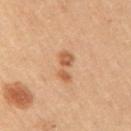Impression: The lesion was tiled from a total-body skin photograph and was not biopsied. Clinical summary: This is a cross-polarized tile. A female subject, aged 43 to 47. This image is a 15 mm lesion crop taken from a total-body photograph. Automated tile analysis of the lesion measured roughly 10 lightness units darker than nearby skin and a lesion-to-skin contrast of about 7 (normalized; higher = more distinct). The lesion is located on the right upper arm. Approximately 4 mm at its widest.